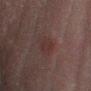Findings:
- workup · imaged on a skin check; not biopsied
- automated metrics · a shape eccentricity near 0.75 and two-axis asymmetry of about 0.2; a border-irregularity index near 2/10 and a color-variation rating of about 1.5/10
- subject · male, about 50 years old
- imaging modality · 15 mm crop, total-body photography
- location · the left lower leg